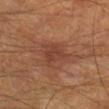imaging modality = total-body-photography crop, ~15 mm field of view
subject = male, aged around 65
site = the right lower leg
lesion diameter = ~3.5 mm (longest diameter)